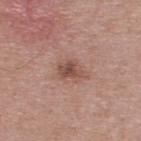Imaged during a routine full-body skin examination; the lesion was not biopsied and no histopathology is available. The patient is a male aged 38 to 42. Longest diameter approximately 3 mm. Located on the upper back. This image is a 15 mm lesion crop taken from a total-body photograph.